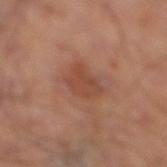Impression:
Part of a total-body skin-imaging series; this lesion was reviewed on a skin check and was not flagged for biopsy.
Image and clinical context:
Measured at roughly 3 mm in maximum diameter. Cropped from a whole-body photographic skin survey; the tile spans about 15 mm. On the leg.